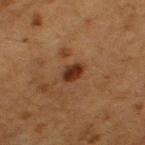{"lighting": "cross-polarized", "site": "left thigh", "lesion_size": {"long_diameter_mm_approx": 2.5}, "image": {"source": "total-body photography crop", "field_of_view_mm": 15}, "patient": {"sex": "female", "age_approx": 50}, "automated_metrics": {"cielab_L": 26, "cielab_a": 19, "cielab_b": 26, "vs_skin_contrast_norm": 11.0, "border_irregularity_0_10": 1.5, "color_variation_0_10": 3.5, "nevus_likeness_0_100": 95}}